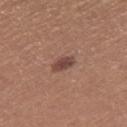workup: no biopsy performed (imaged during a skin exam)
patient: female, aged around 35
tile lighting: white-light illumination
anatomic site: the right thigh
image: ~15 mm tile from a whole-body skin photo
size: about 3 mm
image-analysis metrics: a footprint of about 3.5 mm², a shape eccentricity near 0.85, and a symmetry-axis asymmetry near 0.25